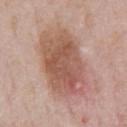Q: Illumination type?
A: white-light
Q: How large is the lesion?
A: about 9 mm
Q: What are the patient's age and sex?
A: male, approximately 50 years of age
Q: Where on the body is the lesion?
A: the chest
Q: How was this image acquired?
A: total-body-photography crop, ~15 mm field of view